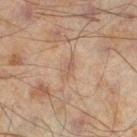workup: total-body-photography surveillance lesion; no biopsy | image-analysis metrics: an area of roughly 2.5 mm², an outline eccentricity of about 0.9 (0 = round, 1 = elongated), and two-axis asymmetry of about 0.3; a mean CIELAB color near L≈45 a*≈14 b*≈24, about 6 CIELAB-L* units darker than the surrounding skin, and a normalized border contrast of about 5 | lighting: cross-polarized | patient: male, in their mid-40s | lesion size: ~2.5 mm (longest diameter) | location: the left lower leg | acquisition: ~15 mm tile from a whole-body skin photo.